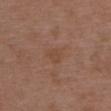Q: Is there a histopathology result?
A: imaged on a skin check; not biopsied
Q: What is the anatomic site?
A: the upper back
Q: Patient demographics?
A: female, aged approximately 30
Q: What did automated image analysis measure?
A: a lesion-to-skin contrast of about 5 (normalized; higher = more distinct)
Q: Illumination type?
A: white-light
Q: How was this image acquired?
A: total-body-photography crop, ~15 mm field of view
Q: What is the lesion's diameter?
A: ~2.5 mm (longest diameter)